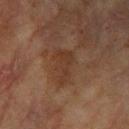workup: catalogued during a skin exam; not biopsied.